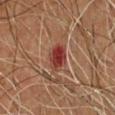The lesion was photographed on a routine skin check and not biopsied; there is no pathology result.
This is a cross-polarized tile.
The lesion is on the chest.
The recorded lesion diameter is about 3.5 mm.
A male patient, aged 63–67.
Cropped from a whole-body photographic skin survey; the tile spans about 15 mm.
Automated tile analysis of the lesion measured a lesion area of about 7 mm² and two-axis asymmetry of about 0.15. And it measured a lesion–skin lightness drop of about 10 and a normalized border contrast of about 10. It also reported a border-irregularity rating of about 1.5/10 and a peripheral color-asymmetry measure near 2.5. The software also gave an automated nevus-likeness rating near 0 out of 100 and a lesion-detection confidence of about 100/100.